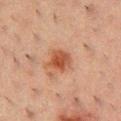The lesion was tiled from a total-body skin photograph and was not biopsied. A close-up tile cropped from a whole-body skin photograph, about 15 mm across. The tile uses cross-polarized illumination. Located on the front of the torso. About 3 mm across. The patient is a male aged approximately 50.